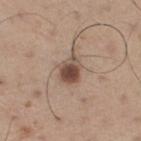The lesion was photographed on a routine skin check and not biopsied; there is no pathology result.
A male patient approximately 65 years of age.
A close-up tile cropped from a whole-body skin photograph, about 15 mm across.
On the right thigh.
This is a white-light tile.